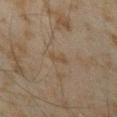<record>
  <biopsy_status>not biopsied; imaged during a skin examination</biopsy_status>
  <image>
    <source>total-body photography crop</source>
    <field_of_view_mm>15</field_of_view_mm>
  </image>
  <patient>
    <sex>male</sex>
    <age_approx>45</age_approx>
  </patient>
  <site>right forearm</site>
</record>